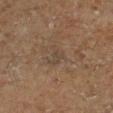workup = imaged on a skin check; not biopsied
automated lesion analysis = an area of roughly 4 mm² and a shape eccentricity near 0.75; a nevus-likeness score of about 0/100 and lesion-presence confidence of about 80/100
tile lighting = cross-polarized illumination
anatomic site = the right lower leg
lesion size = ~3 mm (longest diameter)
subject = male, in their mid- to late 70s
acquisition = 15 mm crop, total-body photography